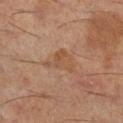Clinical impression:
Recorded during total-body skin imaging; not selected for excision or biopsy.
Image and clinical context:
The patient is a male aged 58 to 62. Located on the right lower leg. The tile uses cross-polarized illumination. A 15 mm crop from a total-body photograph taken for skin-cancer surveillance. The recorded lesion diameter is about 4 mm.